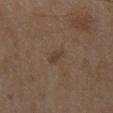  biopsy_status: not biopsied; imaged during a skin examination